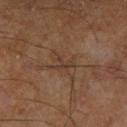biopsy status = total-body-photography surveillance lesion; no biopsy | illumination = cross-polarized illumination | automated lesion analysis = a lesion color around L≈36 a*≈15 b*≈25 in CIELAB, roughly 5 lightness units darker than nearby skin, and a normalized border contrast of about 5; a nevus-likeness score of about 0/100 | subject = male, aged 58 to 62 | site = the right lower leg | image = ~15 mm tile from a whole-body skin photo.